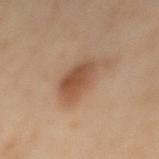Impression: No biopsy was performed on this lesion — it was imaged during a full skin examination and was not determined to be concerning.